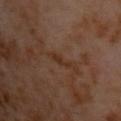Part of a total-body skin-imaging series; this lesion was reviewed on a skin check and was not flagged for biopsy.
The tile uses cross-polarized illumination.
Cropped from a whole-body photographic skin survey; the tile spans about 15 mm.
A male subject, approximately 60 years of age.
Approximately 2.5 mm at its widest.
The lesion is located on the front of the torso.